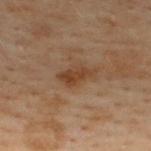Clinical summary: A male subject approximately 50 years of age. The lesion's longest dimension is about 3.5 mm. A region of skin cropped from a whole-body photographic capture, roughly 15 mm wide. The lesion is on the upper back.